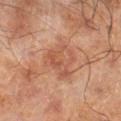Imaged during a routine full-body skin examination; the lesion was not biopsied and no histopathology is available. The patient is a male aged 63 to 67. Automated tile analysis of the lesion measured an area of roughly 12 mm² and a shape eccentricity near 0.7. And it measured a mean CIELAB color near L≈42 a*≈20 b*≈26, a lesion–skin lightness drop of about 6, and a normalized lesion–skin contrast near 5.5. The analysis additionally found a border-irregularity index near 4.5/10, a color-variation rating of about 4/10, and radial color variation of about 1.5. The analysis additionally found a nevus-likeness score of about 0/100. A 15 mm close-up tile from a total-body photography series done for melanoma screening. The tile uses cross-polarized illumination. Longest diameter approximately 5 mm. Located on the left lower leg.